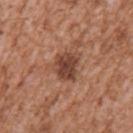Assessment: No biopsy was performed on this lesion — it was imaged during a full skin examination and was not determined to be concerning. Context: The tile uses white-light illumination. A 15 mm crop from a total-body photograph taken for skin-cancer surveillance. From the left upper arm. A male patient, aged 43 to 47.